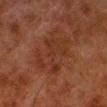Assessment:
The lesion was photographed on a routine skin check and not biopsied; there is no pathology result.
Background:
An algorithmic analysis of the crop reported a footprint of about 15 mm², an eccentricity of roughly 0.65, and a symmetry-axis asymmetry near 0.4. And it measured a border-irregularity rating of about 7/10 and internal color variation of about 2.5 on a 0–10 scale. This is a cross-polarized tile. A 15 mm close-up tile from a total-body photography series done for melanoma screening. The lesion is on the left lower leg. A male patient, in their 80s. The lesion's longest dimension is about 5 mm.